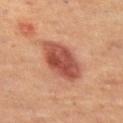{"biopsy_status": "not biopsied; imaged during a skin examination", "image": {"source": "total-body photography crop", "field_of_view_mm": 15}, "patient": {"sex": "female", "age_approx": 40}, "lesion_size": {"long_diameter_mm_approx": 6.0}, "site": "leg", "automated_metrics": {"border_irregularity_0_10": 1.5, "color_variation_0_10": 5.5, "peripheral_color_asymmetry": 2.0, "nevus_likeness_0_100": 100}}